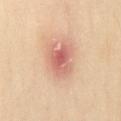notes = no biopsy performed (imaged during a skin exam)
patient = female, about 55 years old
image source = total-body-photography crop, ~15 mm field of view
image-analysis metrics = a lesion area of about 11 mm², an eccentricity of roughly 0.5, and two-axis asymmetry of about 0.3; a border-irregularity rating of about 3.5/10, internal color variation of about 6.5 on a 0–10 scale, and a peripheral color-asymmetry measure near 2; an automated nevus-likeness rating near 0 out of 100 and a lesion-detection confidence of about 100/100
location = the mid back
diameter = ~4 mm (longest diameter)
illumination = cross-polarized